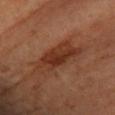The lesion was tiled from a total-body skin photograph and was not biopsied.
Longest diameter approximately 7.5 mm.
A female subject aged 63 to 67.
This is a cross-polarized tile.
The lesion is on the left forearm.
A lesion tile, about 15 mm wide, cut from a 3D total-body photograph.